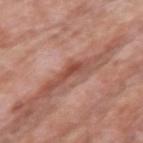biopsy_status: not biopsied; imaged during a skin examination